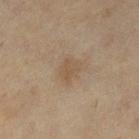Approximately 3 mm at its widest. A roughly 15 mm field-of-view crop from a total-body skin photograph. Automated tile analysis of the lesion measured an average lesion color of about L≈45 a*≈12 b*≈27 (CIELAB), roughly 5 lightness units darker than nearby skin, and a normalized lesion–skin contrast near 5. It also reported a border-irregularity index near 2.5/10, internal color variation of about 2.5 on a 0–10 scale, and a peripheral color-asymmetry measure near 1. This is a cross-polarized tile. A female patient aged around 55. The lesion is located on the left lower leg.